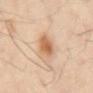Impression: The lesion was photographed on a routine skin check and not biopsied; there is no pathology result. Clinical summary: A 15 mm crop from a total-body photograph taken for skin-cancer surveillance. Automated tile analysis of the lesion measured a lesion area of about 6.5 mm². The software also gave an average lesion color of about L≈63 a*≈21 b*≈36 (CIELAB), roughly 12 lightness units darker than nearby skin, and a lesion-to-skin contrast of about 8 (normalized; higher = more distinct). This is a cross-polarized tile. Approximately 3 mm at its widest. On the mid back. The subject is a male aged 53–57.